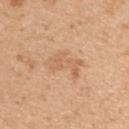Q: Automated lesion metrics?
A: internal color variation of about 2 on a 0–10 scale and a peripheral color-asymmetry measure near 0.5; a detector confidence of about 100 out of 100 that the crop contains a lesion
Q: How was the tile lit?
A: white-light illumination
Q: What are the patient's age and sex?
A: male, approximately 40 years of age
Q: How was this image acquired?
A: ~15 mm crop, total-body skin-cancer survey
Q: Lesion size?
A: ≈4.5 mm
Q: Where on the body is the lesion?
A: the left upper arm